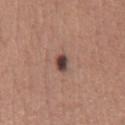{
  "biopsy_status": "not biopsied; imaged during a skin examination",
  "image": {
    "source": "total-body photography crop",
    "field_of_view_mm": 15
  },
  "patient": {
    "sex": "male",
    "age_approx": 45
  },
  "lesion_size": {
    "long_diameter_mm_approx": 2.5
  },
  "site": "front of the torso",
  "automated_metrics": {
    "area_mm2_approx": 3.0,
    "eccentricity": 0.8,
    "shape_asymmetry": 0.2,
    "color_variation_0_10": 4.5,
    "peripheral_color_asymmetry": 1.5,
    "nevus_likeness_0_100": 70,
    "lesion_detection_confidence_0_100": 100
  }
}